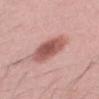Impression:
Imaged during a routine full-body skin examination; the lesion was not biopsied and no histopathology is available.
Background:
Longest diameter approximately 5 mm. A male subject roughly 30 years of age. Cropped from a whole-body photographic skin survey; the tile spans about 15 mm. Automated image analysis of the tile measured a lesion color around L≈55 a*≈25 b*≈24 in CIELAB, about 14 CIELAB-L* units darker than the surrounding skin, and a lesion-to-skin contrast of about 9 (normalized; higher = more distinct). It also reported a border-irregularity rating of about 2/10, a color-variation rating of about 4.5/10, and peripheral color asymmetry of about 1. The lesion is on the mid back. Captured under white-light illumination.